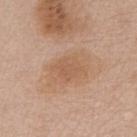Assessment:
Imaged during a routine full-body skin examination; the lesion was not biopsied and no histopathology is available.
Background:
Cropped from a whole-body photographic skin survey; the tile spans about 15 mm. Imaged with white-light lighting. The subject is a female about 70 years old. An algorithmic analysis of the crop reported an outline eccentricity of about 0.7 (0 = round, 1 = elongated) and two-axis asymmetry of about 0.15. And it measured internal color variation of about 1.5 on a 0–10 scale. From the chest.